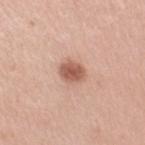| key | value |
|---|---|
| anatomic site | the right upper arm |
| patient | female, approximately 40 years of age |
| image source | ~15 mm tile from a whole-body skin photo |
| diameter | about 3 mm |